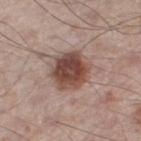Clinical summary:
A male patient aged around 55. A 15 mm close-up extracted from a 3D total-body photography capture. From the right thigh.